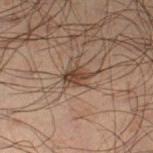notes: catalogued during a skin exam; not biopsied
imaging modality: ~15 mm crop, total-body skin-cancer survey
diameter: ~3 mm (longest diameter)
tile lighting: cross-polarized illumination
automated lesion analysis: a lesion color around L≈32 a*≈13 b*≈22 in CIELAB and roughly 9 lightness units darker than nearby skin; a border-irregularity index near 2/10, a within-lesion color-variation index near 2.5/10, and peripheral color asymmetry of about 1; a classifier nevus-likeness of about 65/100
body site: the left lower leg
subject: male, aged 48 to 52